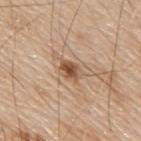This lesion was catalogued during total-body skin photography and was not selected for biopsy.